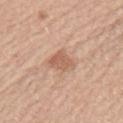The subject is a male in their mid-40s. A 15 mm crop from a total-body photograph taken for skin-cancer surveillance. The tile uses white-light illumination. From the left upper arm.